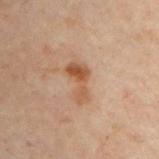This lesion was catalogued during total-body skin photography and was not selected for biopsy.
A roughly 15 mm field-of-view crop from a total-body skin photograph.
Automated tile analysis of the lesion measured an area of roughly 6 mm², an eccentricity of roughly 0.9, and a symmetry-axis asymmetry near 0.55.
Measured at roughly 4 mm in maximum diameter.
A male subject, roughly 50 years of age.
This is a cross-polarized tile.
The lesion is on the chest.